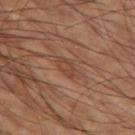Case summary:
• biopsy status — imaged on a skin check; not biopsied
• lighting — cross-polarized illumination
• image-analysis metrics — a symmetry-axis asymmetry near 0.25; a lesion color around L≈42 a*≈21 b*≈28 in CIELAB, about 6 CIELAB-L* units darker than the surrounding skin, and a normalized border contrast of about 5.5; border irregularity of about 2 on a 0–10 scale, internal color variation of about 2 on a 0–10 scale, and peripheral color asymmetry of about 0.5
• size — ≈2.5 mm
• subject — male, roughly 60 years of age
• location — the left thigh
• acquisition — ~15 mm crop, total-body skin-cancer survey